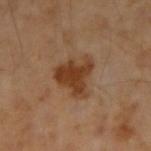biopsy_status: not biopsied; imaged during a skin examination
patient:
  sex: male
  age_approx: 45
image:
  source: total-body photography crop
  field_of_view_mm: 15
site: right forearm